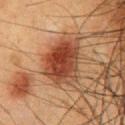This lesion was catalogued during total-body skin photography and was not selected for biopsy. The total-body-photography lesion software estimated an area of roughly 19 mm², an outline eccentricity of about 0.65 (0 = round, 1 = elongated), and a shape-asymmetry score of about 0.2 (0 = symmetric). Imaged with cross-polarized lighting. On the chest. A male patient, aged 48 to 52. A 15 mm crop from a total-body photograph taken for skin-cancer surveillance.